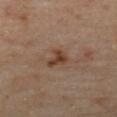<lesion>
  <biopsy_status>not biopsied; imaged during a skin examination</biopsy_status>
  <lesion_size>
    <long_diameter_mm_approx>2.5</long_diameter_mm_approx>
  </lesion_size>
  <lighting>cross-polarized</lighting>
  <patient>
    <sex>female</sex>
    <age_approx>60</age_approx>
  </patient>
  <site>back</site>
  <automated_metrics>
    <area_mm2_approx>4.5</area_mm2_approx>
    <eccentricity>0.35</eccentricity>
    <shape_asymmetry>0.4</shape_asymmetry>
    <cielab_L>40</cielab_L>
    <cielab_a>18</cielab_a>
    <cielab_b>28</cielab_b>
    <vs_skin_darker_L>10.0</vs_skin_darker_L>
    <vs_skin_contrast_norm>8.5</vs_skin_contrast_norm>
  </automated_metrics>
  <image>
    <source>total-body photography crop</source>
    <field_of_view_mm>15</field_of_view_mm>
  </image>
</lesion>